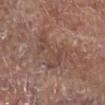Q: Was a biopsy performed?
A: imaged on a skin check; not biopsied
Q: What is the anatomic site?
A: the right lower leg
Q: What are the patient's age and sex?
A: male, aged 68 to 72
Q: Illumination type?
A: white-light illumination
Q: Automated lesion metrics?
A: a classifier nevus-likeness of about 0/100 and a detector confidence of about 90 out of 100 that the crop contains a lesion
Q: Lesion size?
A: about 6.5 mm
Q: How was this image acquired?
A: 15 mm crop, total-body photography